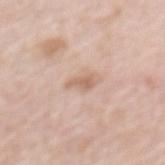biopsy status: no biopsy performed (imaged during a skin exam)
body site: the mid back
image source: ~15 mm tile from a whole-body skin photo
lesion size: ~2.5 mm (longest diameter)
subject: female, in their mid-60s
lighting: white-light